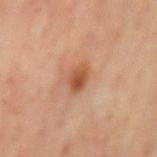Impression: No biopsy was performed on this lesion — it was imaged during a full skin examination and was not determined to be concerning. Clinical summary: This is a cross-polarized tile. On the mid back. A male patient aged 68 to 72. This image is a 15 mm lesion crop taken from a total-body photograph.